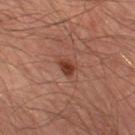Q: Was a biopsy performed?
A: imaged on a skin check; not biopsied
Q: What is the imaging modality?
A: total-body-photography crop, ~15 mm field of view
Q: Lesion location?
A: the left thigh
Q: Patient demographics?
A: male, approximately 55 years of age
Q: What lighting was used for the tile?
A: cross-polarized illumination
Q: What is the lesion's diameter?
A: ~2 mm (longest diameter)